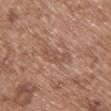Q: Was a biopsy performed?
A: catalogued during a skin exam; not biopsied
Q: What kind of image is this?
A: ~15 mm tile from a whole-body skin photo
Q: What is the anatomic site?
A: the front of the torso
Q: Who is the patient?
A: female, about 75 years old